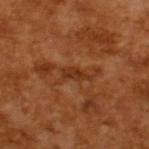follow-up: catalogued during a skin exam; not biopsied | lighting: cross-polarized | patient: male, in their mid-60s | image source: 15 mm crop, total-body photography | diameter: ~3.5 mm (longest diameter).